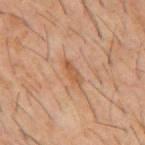| feature | finding |
|---|---|
| notes | no biopsy performed (imaged during a skin exam) |
| image source | ~15 mm tile from a whole-body skin photo |
| body site | the mid back |
| patient | male, aged approximately 60 |
| size | ≈3.5 mm |